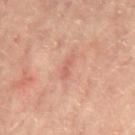Q: Is there a histopathology result?
A: no biopsy performed (imaged during a skin exam)
Q: How was the tile lit?
A: cross-polarized
Q: What is the imaging modality?
A: 15 mm crop, total-body photography
Q: What is the lesion's diameter?
A: about 2.5 mm
Q: Patient demographics?
A: male, aged approximately 65
Q: Automated lesion metrics?
A: an area of roughly 2.5 mm², an outline eccentricity of about 0.9 (0 = round, 1 = elongated), and a shape-asymmetry score of about 0.5 (0 = symmetric); a color-variation rating of about 0/10 and peripheral color asymmetry of about 0
Q: Lesion location?
A: the mid back